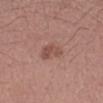{
  "biopsy_status": "not biopsied; imaged during a skin examination",
  "automated_metrics": {
    "area_mm2_approx": 5.0,
    "eccentricity": 0.3,
    "shape_asymmetry": 0.35,
    "cielab_L": 50,
    "cielab_a": 22,
    "cielab_b": 25,
    "vs_skin_darker_L": 8.0,
    "vs_skin_contrast_norm": 6.0,
    "nevus_likeness_0_100": 5,
    "lesion_detection_confidence_0_100": 100
  },
  "lesion_size": {
    "long_diameter_mm_approx": 2.5
  },
  "site": "arm",
  "patient": {
    "sex": "male",
    "age_approx": 45
  },
  "image": {
    "source": "total-body photography crop",
    "field_of_view_mm": 15
  }
}